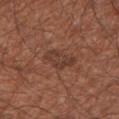{"biopsy_status": "not biopsied; imaged during a skin examination", "automated_metrics": {"cielab_L": 38, "cielab_a": 21, "cielab_b": 26, "vs_skin_darker_L": 7.0, "nevus_likeness_0_100": 0}, "lighting": "white-light", "image": {"source": "total-body photography crop", "field_of_view_mm": 15}, "patient": {"sex": "male", "age_approx": 65}, "site": "right upper arm", "lesion_size": {"long_diameter_mm_approx": 3.5}}